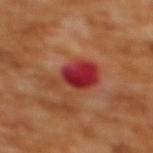Impression: The lesion was tiled from a total-body skin photograph and was not biopsied. Background: The subject is a female aged 53 to 57. A roughly 15 mm field-of-view crop from a total-body skin photograph. From the upper back. This is a cross-polarized tile. Approximately 6.5 mm at its widest. The total-body-photography lesion software estimated a lesion area of about 12 mm².